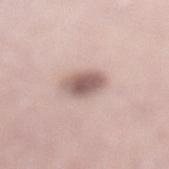The lesion was tiled from a total-body skin photograph and was not biopsied. Cropped from a whole-body photographic skin survey; the tile spans about 15 mm. The tile uses white-light illumination. The lesion is located on the leg. Approximately 3 mm at its widest. The subject is a female aged approximately 40. Automated image analysis of the tile measured a lesion area of about 8.5 mm², an outline eccentricity of about 0.5 (0 = round, 1 = elongated), and a shape-asymmetry score of about 0.15 (0 = symmetric). The software also gave a border-irregularity rating of about 1.5/10, a color-variation rating of about 3.5/10, and a peripheral color-asymmetry measure near 1.